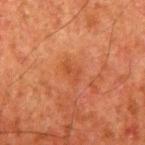Q: Is there a histopathology result?
A: catalogued during a skin exam; not biopsied
Q: Who is the patient?
A: male, approximately 80 years of age
Q: What lighting was used for the tile?
A: cross-polarized
Q: What kind of image is this?
A: ~15 mm tile from a whole-body skin photo
Q: Where on the body is the lesion?
A: the arm
Q: Automated lesion metrics?
A: an outline eccentricity of about 0.8 (0 = round, 1 = elongated); a mean CIELAB color near L≈39 a*≈25 b*≈33 and a normalized border contrast of about 4.5; border irregularity of about 3.5 on a 0–10 scale and radial color variation of about 1; a classifier nevus-likeness of about 0/100 and a lesion-detection confidence of about 100/100